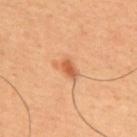  biopsy_status: not biopsied; imaged during a skin examination
  site: back
  automated_metrics:
    area_mm2_approx: 4.0
    eccentricity: 0.8
    shape_asymmetry: 0.3
    cielab_L: 58
    cielab_a: 27
    cielab_b: 39
    vs_skin_darker_L: 11.0
    vs_skin_contrast_norm: 7.0
    nevus_likeness_0_100: 90
    lesion_detection_confidence_0_100: 100
  patient:
    sex: male
    age_approx: 65
  image:
    source: total-body photography crop
    field_of_view_mm: 15
  lesion_size:
    long_diameter_mm_approx: 3.0
  lighting: cross-polarized